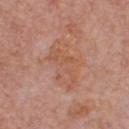Q: Is there a histopathology result?
A: total-body-photography surveillance lesion; no biopsy
Q: What kind of image is this?
A: ~15 mm crop, total-body skin-cancer survey
Q: Illumination type?
A: white-light
Q: What are the patient's age and sex?
A: male, approximately 60 years of age
Q: What did automated image analysis measure?
A: a symmetry-axis asymmetry near 0.35
Q: What is the lesion's diameter?
A: about 6 mm
Q: Where on the body is the lesion?
A: the chest